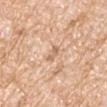Impression: This lesion was catalogued during total-body skin photography and was not selected for biopsy. Background: A region of skin cropped from a whole-body photographic capture, roughly 15 mm wide. About 2.5 mm across. This is a white-light tile. On the right upper arm. The subject is a male aged 58–62. The lesion-visualizer software estimated an area of roughly 2.5 mm² and an outline eccentricity of about 0.9 (0 = round, 1 = elongated).